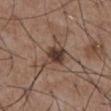Recorded during total-body skin imaging; not selected for excision or biopsy.
This image is a 15 mm lesion crop taken from a total-body photograph.
A male patient, aged approximately 55.
An algorithmic analysis of the crop reported an eccentricity of roughly 0.8. The software also gave a mean CIELAB color near L≈39 a*≈16 b*≈23, about 12 CIELAB-L* units darker than the surrounding skin, and a lesion-to-skin contrast of about 10 (normalized; higher = more distinct). The analysis additionally found border irregularity of about 4 on a 0–10 scale. And it measured an automated nevus-likeness rating near 95 out of 100.
The lesion is on the abdomen.
Longest diameter approximately 4 mm.